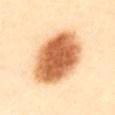Impression: The lesion was photographed on a routine skin check and not biopsied; there is no pathology result. Context: The total-body-photography lesion software estimated an area of roughly 33 mm², a shape eccentricity near 0.6, and a shape-asymmetry score of about 0.1 (0 = symmetric). The analysis additionally found an average lesion color of about L≈67 a*≈25 b*≈43 (CIELAB), a lesion–skin lightness drop of about 22, and a normalized lesion–skin contrast near 12. On the abdomen. A close-up tile cropped from a whole-body skin photograph, about 15 mm across. The patient is a female aged around 60. The tile uses cross-polarized illumination. Approximately 7.5 mm at its widest.